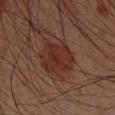Findings:
– biopsy status — total-body-photography surveillance lesion; no biopsy
– lighting — cross-polarized
– diameter — about 4.5 mm
– anatomic site — the left forearm
– image — 15 mm crop, total-body photography
– patient — male, roughly 50 years of age